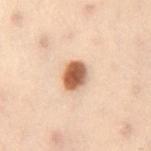| feature | finding |
|---|---|
| biopsy status | no biopsy performed (imaged during a skin exam) |
| site | the right thigh |
| imaging modality | 15 mm crop, total-body photography |
| subject | female, approximately 50 years of age |
| automated lesion analysis | a lesion area of about 7 mm², an outline eccentricity of about 0.7 (0 = round, 1 = elongated), and a symmetry-axis asymmetry near 0.2; a lesion color around L≈51 a*≈20 b*≈30 in CIELAB, a lesion–skin lightness drop of about 18, and a normalized lesion–skin contrast near 12 |